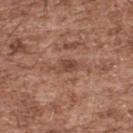Findings:
• biopsy status · imaged on a skin check; not biopsied
• imaging modality · total-body-photography crop, ~15 mm field of view
• lesion size · about 3 mm
• tile lighting · white-light
• subject · male, aged approximately 55
• location · the upper back